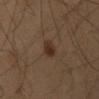Findings:
* biopsy status — no biopsy performed (imaged during a skin exam)
* lesion size — about 3.5 mm
* lighting — cross-polarized illumination
* image source — 15 mm crop, total-body photography
* patient — male, aged 53 to 57
* automated lesion analysis — an area of roughly 4.5 mm² and an outline eccentricity of about 0.95 (0 = round, 1 = elongated); a lesion color around L≈29 a*≈15 b*≈24 in CIELAB, roughly 8 lightness units darker than nearby skin, and a normalized border contrast of about 8
* body site — the mid back